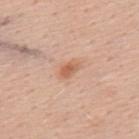Impression: This lesion was catalogued during total-body skin photography and was not selected for biopsy. Background: The total-body-photography lesion software estimated an eccentricity of roughly 0.8 and a symmetry-axis asymmetry near 0.25. And it measured a lesion color around L≈61 a*≈24 b*≈33 in CIELAB and a lesion–skin lightness drop of about 10. It also reported a nevus-likeness score of about 80/100 and a detector confidence of about 100 out of 100 that the crop contains a lesion. The tile uses white-light illumination. A male subject, aged 53 to 57. The lesion is located on the upper back. Cropped from a total-body skin-imaging series; the visible field is about 15 mm.